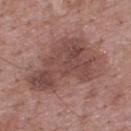<lesion>
<biopsy_status>not biopsied; imaged during a skin examination</biopsy_status>
<automated_metrics>
  <border_irregularity_0_10>5.0</border_irregularity_0_10>
  <color_variation_0_10>5.0</color_variation_0_10>
  <peripheral_color_asymmetry>2.0</peripheral_color_asymmetry>
</automated_metrics>
<site>upper back</site>
<image>
  <source>total-body photography crop</source>
  <field_of_view_mm>15</field_of_view_mm>
</image>
<lighting>white-light</lighting>
<lesion_size>
  <long_diameter_mm_approx>9.5</long_diameter_mm_approx>
</lesion_size>
<patient>
  <sex>male</sex>
  <age_approx>70</age_approx>
</patient>
</lesion>